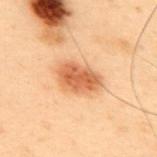<lesion>
<biopsy_status>not biopsied; imaged during a skin examination</biopsy_status>
<lighting>cross-polarized</lighting>
<image>
  <source>total-body photography crop</source>
  <field_of_view_mm>15</field_of_view_mm>
</image>
<site>upper back</site>
<patient>
  <sex>male</sex>
  <age_approx>55</age_approx>
</patient>
<lesion_size>
  <long_diameter_mm_approx>4.5</long_diameter_mm_approx>
</lesion_size>
<automated_metrics>
  <cielab_L>51</cielab_L>
  <cielab_a>23</cielab_a>
  <cielab_b>34</cielab_b>
  <vs_skin_darker_L>12.0</vs_skin_darker_L>
  <vs_skin_contrast_norm>8.5</vs_skin_contrast_norm>
  <border_irregularity_0_10>2.0</border_irregularity_0_10>
  <peripheral_color_asymmetry>1.0</peripheral_color_asymmetry>
</automated_metrics>
</lesion>